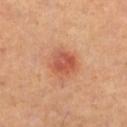Q: How large is the lesion?
A: ≈3.5 mm
Q: Illumination type?
A: cross-polarized
Q: Where on the body is the lesion?
A: the leg
Q: How was this image acquired?
A: total-body-photography crop, ~15 mm field of view
Q: What did automated image analysis measure?
A: an area of roughly 8 mm² and a shape-asymmetry score of about 0.2 (0 = symmetric); border irregularity of about 2 on a 0–10 scale, a color-variation rating of about 4.5/10, and peripheral color asymmetry of about 1.5
Q: Who is the patient?
A: female, approximately 40 years of age